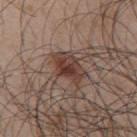Impression: Part of a total-body skin-imaging series; this lesion was reviewed on a skin check and was not flagged for biopsy. Context: Imaged with white-light lighting. From the upper back. Automated tile analysis of the lesion measured a within-lesion color-variation index near 6/10. The analysis additionally found a classifier nevus-likeness of about 95/100. Measured at roughly 5 mm in maximum diameter. A lesion tile, about 15 mm wide, cut from a 3D total-body photograph. A male subject aged 48–52.